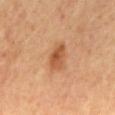Clinical impression: This lesion was catalogued during total-body skin photography and was not selected for biopsy. Context: A region of skin cropped from a whole-body photographic capture, roughly 15 mm wide. A male subject aged around 60. The lesion is located on the mid back. About 3 mm across.